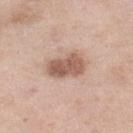| feature | finding |
|---|---|
| follow-up | total-body-photography surveillance lesion; no biopsy |
| subject | female, aged approximately 55 |
| image-analysis metrics | two-axis asymmetry of about 0.15; a mean CIELAB color near L≈58 a*≈19 b*≈27, about 13 CIELAB-L* units darker than the surrounding skin, and a normalized border contrast of about 8.5; a classifier nevus-likeness of about 45/100 and a lesion-detection confidence of about 100/100 |
| lesion diameter | ~4.5 mm (longest diameter) |
| imaging modality | ~15 mm tile from a whole-body skin photo |
| location | the leg |